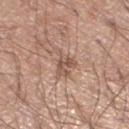workup: total-body-photography surveillance lesion; no biopsy
lesion diameter: ≈4 mm
illumination: white-light illumination
image-analysis metrics: a footprint of about 5.5 mm², an eccentricity of roughly 0.75, and two-axis asymmetry of about 0.5; a mean CIELAB color near L≈54 a*≈19 b*≈27, about 10 CIELAB-L* units darker than the surrounding skin, and a normalized border contrast of about 6.5
patient: male, aged 18–22
body site: the left lower leg
image source: ~15 mm crop, total-body skin-cancer survey